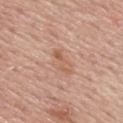biopsy status: no biopsy performed (imaged during a skin exam) | body site: the upper back | image-analysis metrics: a lesion area of about 4 mm², a shape eccentricity near 0.9, and a shape-asymmetry score of about 0.4 (0 = symmetric); an average lesion color of about L≈59 a*≈22 b*≈31 (CIELAB), roughly 7 lightness units darker than nearby skin, and a lesion-to-skin contrast of about 6 (normalized; higher = more distinct); border irregularity of about 5 on a 0–10 scale, a within-lesion color-variation index near 0.5/10, and peripheral color asymmetry of about 0; a nevus-likeness score of about 0/100 | acquisition: 15 mm crop, total-body photography | illumination: white-light | subject: female, aged around 65 | lesion size: ~3.5 mm (longest diameter).